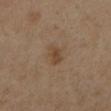notes — catalogued during a skin exam; not biopsied | image — 15 mm crop, total-body photography | patient — male, aged approximately 65 | lesion diameter — ~3 mm (longest diameter) | anatomic site — the left lower leg | tile lighting — cross-polarized illumination.